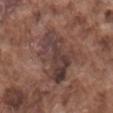Case summary:
* follow-up: imaged on a skin check; not biopsied
* size: about 7 mm
* automated metrics: an automated nevus-likeness rating near 0 out of 100 and a detector confidence of about 70 out of 100 that the crop contains a lesion
* tile lighting: white-light
* anatomic site: the mid back
* patient: male, in their mid- to late 70s
* image: ~15 mm crop, total-body skin-cancer survey